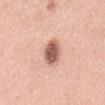{"biopsy_status": "not biopsied; imaged during a skin examination", "site": "abdomen", "image": {"source": "total-body photography crop", "field_of_view_mm": 15}, "patient": {"sex": "male", "age_approx": 60}, "lighting": "white-light"}